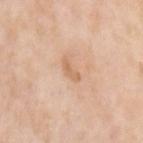Clinical impression: Recorded during total-body skin imaging; not selected for excision or biopsy. Clinical summary: Imaged with white-light lighting. The lesion is on the left upper arm. The recorded lesion diameter is about 2.5 mm. Automated tile analysis of the lesion measured an area of roughly 2 mm², a shape eccentricity near 0.9, and two-axis asymmetry of about 0.55. It also reported border irregularity of about 5.5 on a 0–10 scale, internal color variation of about 0 on a 0–10 scale, and a peripheral color-asymmetry measure near 0. It also reported a nevus-likeness score of about 0/100 and a detector confidence of about 100 out of 100 that the crop contains a lesion. A female subject, aged approximately 60. A close-up tile cropped from a whole-body skin photograph, about 15 mm across.